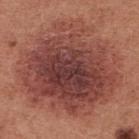workup = catalogued during a skin exam; not biopsied | subject = female, aged around 35 | size = about 11 mm | anatomic site = the chest | image source = total-body-photography crop, ~15 mm field of view.